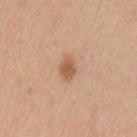Q: Was this lesion biopsied?
A: no biopsy performed (imaged during a skin exam)
Q: Patient demographics?
A: female, roughly 45 years of age
Q: What kind of image is this?
A: 15 mm crop, total-body photography
Q: Automated lesion metrics?
A: a lesion area of about 4.5 mm², an eccentricity of roughly 0.7, and a symmetry-axis asymmetry near 0.2; a border-irregularity rating of about 1.5/10, a color-variation rating of about 2.5/10, and a peripheral color-asymmetry measure near 1
Q: How large is the lesion?
A: ~2.5 mm (longest diameter)
Q: Where on the body is the lesion?
A: the left upper arm